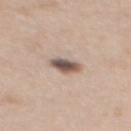{"biopsy_status": "not biopsied; imaged during a skin examination", "patient": {"sex": "female", "age_approx": 40}, "site": "mid back", "image": {"source": "total-body photography crop", "field_of_view_mm": 15}}